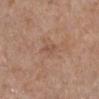Impression:
No biopsy was performed on this lesion — it was imaged during a full skin examination and was not determined to be concerning.
Image and clinical context:
A lesion tile, about 15 mm wide, cut from a 3D total-body photograph. Longest diameter approximately 2.5 mm. The total-body-photography lesion software estimated an area of roughly 2.5 mm², a shape eccentricity near 0.8, and a shape-asymmetry score of about 0.35 (0 = symmetric). This is a white-light tile. From the right lower leg. A female subject, roughly 45 years of age.